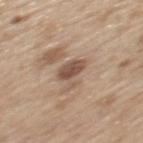Notes:
– notes: catalogued during a skin exam; not biopsied
– tile lighting: white-light
– image source: total-body-photography crop, ~15 mm field of view
– subject: male, roughly 70 years of age
– location: the mid back
– lesion diameter: about 3 mm